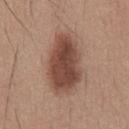Context:
The subject is a male about 35 years old. Imaged with white-light lighting. On the chest. The recorded lesion diameter is about 7 mm. This image is a 15 mm lesion crop taken from a total-body photograph.